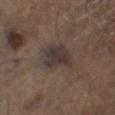{
  "biopsy_status": "not biopsied; imaged during a skin examination",
  "lighting": "cross-polarized",
  "image": {
    "source": "total-body photography crop",
    "field_of_view_mm": 15
  },
  "site": "left lower leg",
  "lesion_size": {
    "long_diameter_mm_approx": 3.5
  },
  "patient": {
    "sex": "male",
    "age_approx": 65
  }
}